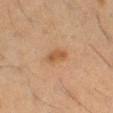The lesion was tiled from a total-body skin photograph and was not biopsied. The patient is a male aged approximately 60. A region of skin cropped from a whole-body photographic capture, roughly 15 mm wide. From the left thigh.